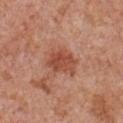Q: Was this lesion biopsied?
A: no biopsy performed (imaged during a skin exam)
Q: What are the patient's age and sex?
A: male, aged 63–67
Q: Illumination type?
A: white-light illumination
Q: Where on the body is the lesion?
A: the front of the torso
Q: What kind of image is this?
A: total-body-photography crop, ~15 mm field of view
Q: What did automated image analysis measure?
A: about 10 CIELAB-L* units darker than the surrounding skin and a normalized border contrast of about 7.5; a classifier nevus-likeness of about 90/100 and a detector confidence of about 100 out of 100 that the crop contains a lesion